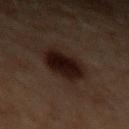follow-up = imaged on a skin check; not biopsied | site = the left upper arm | image = ~15 mm tile from a whole-body skin photo | patient = male, approximately 70 years of age | diameter = ~4.5 mm (longest diameter) | tile lighting = cross-polarized | TBP lesion metrics = a nevus-likeness score of about 100/100 and a lesion-detection confidence of about 100/100.